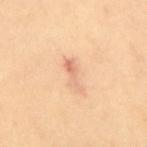The lesion was tiled from a total-body skin photograph and was not biopsied.
The subject is a female approximately 45 years of age.
Located on the upper back.
A region of skin cropped from a whole-body photographic capture, roughly 15 mm wide.